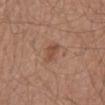{"biopsy_status": "not biopsied; imaged during a skin examination", "site": "abdomen", "image": {"source": "total-body photography crop", "field_of_view_mm": 15}, "patient": {"sex": "male", "age_approx": 65}}